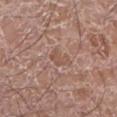Recorded during total-body skin imaging; not selected for excision or biopsy. Automated tile analysis of the lesion measured a lesion–skin lightness drop of about 6 and a normalized border contrast of about 5. A male subject aged 58–62. Measured at roughly 3 mm in maximum diameter. The lesion is on the leg. A lesion tile, about 15 mm wide, cut from a 3D total-body photograph. This is a white-light tile.